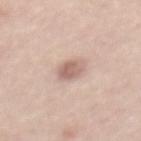No biopsy was performed on this lesion — it was imaged during a full skin examination and was not determined to be concerning. The lesion is located on the mid back. A male subject aged approximately 60. A region of skin cropped from a whole-body photographic capture, roughly 15 mm wide.